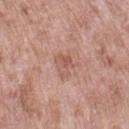Case summary:
– biopsy status · no biopsy performed (imaged during a skin exam)
– location · the left upper arm
– automated metrics · a peripheral color-asymmetry measure near 1.5; a classifier nevus-likeness of about 0/100 and a detector confidence of about 100 out of 100 that the crop contains a lesion
– acquisition · 15 mm crop, total-body photography
– illumination · white-light
– lesion size · ≈3 mm
– subject · female, approximately 70 years of age